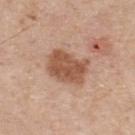{
  "biopsy_status": "not biopsied; imaged during a skin examination",
  "patient": {
    "sex": "male",
    "age_approx": 60
  },
  "image": {
    "source": "total-body photography crop",
    "field_of_view_mm": 15
  },
  "lesion_size": {
    "long_diameter_mm_approx": 5.0
  },
  "lighting": "white-light",
  "site": "upper back"
}